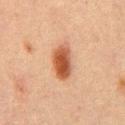Impression:
Recorded during total-body skin imaging; not selected for excision or biopsy.